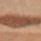Captured during whole-body skin photography for melanoma surveillance; the lesion was not biopsied. Longest diameter approximately 20.5 mm. The patient is a female in their 60s. Located on the upper back. Cropped from a total-body skin-imaging series; the visible field is about 15 mm.